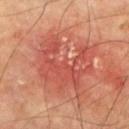The lesion was tiled from a total-body skin photograph and was not biopsied. Automated image analysis of the tile measured a nevus-likeness score of about 0/100 and a lesion-detection confidence of about 100/100. A male subject aged around 75. A lesion tile, about 15 mm wide, cut from a 3D total-body photograph. On the right upper arm. Measured at roughly 7 mm in maximum diameter.